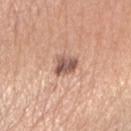This lesion was catalogued during total-body skin photography and was not selected for biopsy.
The total-body-photography lesion software estimated a lesion area of about 5.5 mm², an eccentricity of roughly 0.3, and a symmetry-axis asymmetry near 0.3. The software also gave a mean CIELAB color near L≈55 a*≈21 b*≈26 and roughly 14 lightness units darker than nearby skin.
Captured under white-light illumination.
Cropped from a total-body skin-imaging series; the visible field is about 15 mm.
A female subject aged 58–62.
The lesion is on the right upper arm.